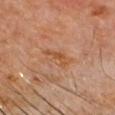Clinical impression:
Imaged during a routine full-body skin examination; the lesion was not biopsied and no histopathology is available.